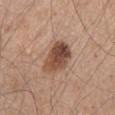A male subject, aged 43 to 47. This is a white-light tile. Cropped from a whole-body photographic skin survey; the tile spans about 15 mm. Automated image analysis of the tile measured a lesion area of about 11 mm². And it measured an average lesion color of about L≈48 a*≈20 b*≈28 (CIELAB), roughly 14 lightness units darker than nearby skin, and a normalized border contrast of about 10. The analysis additionally found a border-irregularity index near 2.5/10, internal color variation of about 8 on a 0–10 scale, and peripheral color asymmetry of about 3. The lesion is on the mid back.